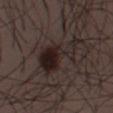Q: Is there a histopathology result?
A: catalogued during a skin exam; not biopsied